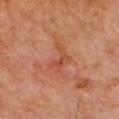Q: Is there a histopathology result?
A: no biopsy performed (imaged during a skin exam)
Q: Patient demographics?
A: male, aged around 60
Q: How was this image acquired?
A: 15 mm crop, total-body photography
Q: Illumination type?
A: cross-polarized illumination
Q: Automated lesion metrics?
A: a nevus-likeness score of about 0/100 and a detector confidence of about 100 out of 100 that the crop contains a lesion
Q: What is the anatomic site?
A: the head or neck
Q: How large is the lesion?
A: ≈4 mm